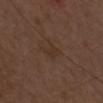Part of a total-body skin-imaging series; this lesion was reviewed on a skin check and was not flagged for biopsy. From the head or neck. The tile uses white-light illumination. A lesion tile, about 15 mm wide, cut from a 3D total-body photograph. Automated image analysis of the tile measured a lesion area of about 3.5 mm², an eccentricity of roughly 0.85, and a symmetry-axis asymmetry near 0.25. And it measured border irregularity of about 2.5 on a 0–10 scale, a within-lesion color-variation index near 0.5/10, and peripheral color asymmetry of about 0. The software also gave an automated nevus-likeness rating near 5 out of 100. A female patient aged 28–32. The lesion's longest dimension is about 3 mm.